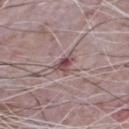Part of a total-body skin-imaging series; this lesion was reviewed on a skin check and was not flagged for biopsy. A male subject, about 65 years old. On the chest. Imaged with white-light lighting. Cropped from a whole-body photographic skin survey; the tile spans about 15 mm.